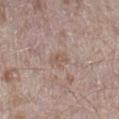Q: Was this lesion biopsied?
A: imaged on a skin check; not biopsied
Q: How was the tile lit?
A: white-light
Q: Patient demographics?
A: male, in their mid- to late 40s
Q: What kind of image is this?
A: ~15 mm tile from a whole-body skin photo
Q: Where on the body is the lesion?
A: the right lower leg